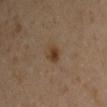| key | value |
|---|---|
| notes | imaged on a skin check; not biopsied |
| image | 15 mm crop, total-body photography |
| lesion size | ≈2.5 mm |
| tile lighting | cross-polarized illumination |
| patient | male, aged approximately 45 |
| site | the right upper arm |
| TBP lesion metrics | border irregularity of about 2 on a 0–10 scale, internal color variation of about 4 on a 0–10 scale, and a peripheral color-asymmetry measure near 1.5; lesion-presence confidence of about 100/100 |